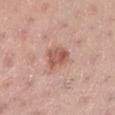No biopsy was performed on this lesion — it was imaged during a full skin examination and was not determined to be concerning.
On the left lower leg.
A region of skin cropped from a whole-body photographic capture, roughly 15 mm wide.
This is a white-light tile.
A female subject in their mid-50s.
The total-body-photography lesion software estimated a border-irregularity index near 2.5/10 and internal color variation of about 5 on a 0–10 scale. It also reported a nevus-likeness score of about 80/100 and a lesion-detection confidence of about 100/100.
Measured at roughly 3.5 mm in maximum diameter.